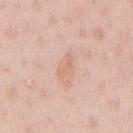Assessment: Recorded during total-body skin imaging; not selected for excision or biopsy.